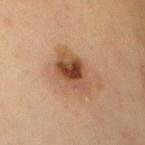This lesion was catalogued during total-body skin photography and was not selected for biopsy. Located on the chest. A female patient aged approximately 60. Cropped from a whole-body photographic skin survey; the tile spans about 15 mm.